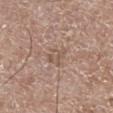  lesion_size:
    long_diameter_mm_approx: 2.5
  lighting: white-light
  automated_metrics:
    area_mm2_approx: 4.0
    eccentricity: 0.65
    cielab_L: 54
    cielab_a: 17
    cielab_b: 26
    vs_skin_darker_L: 7.0
    vs_skin_contrast_norm: 5.0
    nevus_likeness_0_100: 0
  image:
    source: total-body photography crop
    field_of_view_mm: 15
  site: left lower leg
  patient:
    sex: male
    age_approx: 65